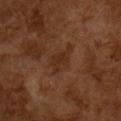Imaged during a routine full-body skin examination; the lesion was not biopsied and no histopathology is available. The lesion-visualizer software estimated a lesion area of about 7 mm². It also reported an automated nevus-likeness rating near 0 out of 100 and lesion-presence confidence of about 100/100. Imaged with cross-polarized lighting. A lesion tile, about 15 mm wide, cut from a 3D total-body photograph. Measured at roughly 4 mm in maximum diameter. The patient is a male roughly 65 years of age.